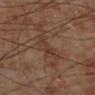Clinical impression:
This lesion was catalogued during total-body skin photography and was not selected for biopsy.
Context:
The tile uses cross-polarized illumination. The lesion is located on the left lower leg. A male subject in their 70s. A 15 mm close-up tile from a total-body photography series done for melanoma screening. The lesion-visualizer software estimated an area of roughly 5.5 mm², an outline eccentricity of about 0.9 (0 = round, 1 = elongated), and a symmetry-axis asymmetry near 0.5. It also reported an average lesion color of about L≈35 a*≈18 b*≈26 (CIELAB) and a normalized border contrast of about 6. It also reported an automated nevus-likeness rating near 0 out of 100 and lesion-presence confidence of about 85/100.